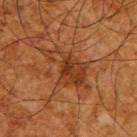| field | value |
|---|---|
| workup | no biopsy performed (imaged during a skin exam) |
| patient | male, aged around 65 |
| lesion size | ~7 mm (longest diameter) |
| site | the upper back |
| acquisition | ~15 mm crop, total-body skin-cancer survey |
| illumination | cross-polarized |